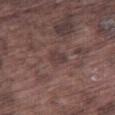Clinical impression: This lesion was catalogued during total-body skin photography and was not selected for biopsy. Background: The recorded lesion diameter is about 2.5 mm. A male patient, in their mid- to late 70s. Captured under white-light illumination. The lesion is located on the leg. This image is a 15 mm lesion crop taken from a total-body photograph.